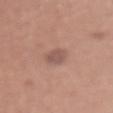This lesion was catalogued during total-body skin photography and was not selected for biopsy. Imaged with white-light lighting. A 15 mm crop from a total-body photograph taken for skin-cancer surveillance. Located on the abdomen. About 3 mm across. The subject is a male approximately 45 years of age.